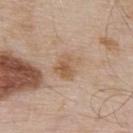| feature | finding |
|---|---|
| follow-up | imaged on a skin check; not biopsied |
| anatomic site | the upper back |
| acquisition | ~15 mm tile from a whole-body skin photo |
| patient | male, in their mid-50s |